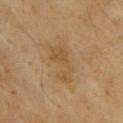The lesion was photographed on a routine skin check and not biopsied; there is no pathology result. An algorithmic analysis of the crop reported a within-lesion color-variation index near 2/10 and peripheral color asymmetry of about 0.5. Cropped from a total-body skin-imaging series; the visible field is about 15 mm. Located on the chest. The patient is a male about 65 years old. The tile uses cross-polarized illumination.